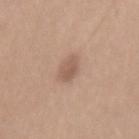  biopsy_status: not biopsied; imaged during a skin examination
  site: back
  automated_metrics:
    area_mm2_approx: 4.0
    eccentricity: 0.65
    border_irregularity_0_10: 1.5
    color_variation_0_10: 2.5
    peripheral_color_asymmetry: 1.0
  patient:
    sex: female
    age_approx: 35
  lesion_size:
    long_diameter_mm_approx: 2.5
  image:
    source: total-body photography crop
    field_of_view_mm: 15
  lighting: white-light